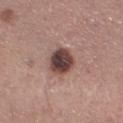biopsy status = catalogued during a skin exam; not biopsied | lesion diameter = about 3.5 mm | image-analysis metrics = border irregularity of about 1 on a 0–10 scale and peripheral color asymmetry of about 1.5; an automated nevus-likeness rating near 30 out of 100 and a detector confidence of about 100 out of 100 that the crop contains a lesion | site = the left lower leg | image = ~15 mm crop, total-body skin-cancer survey | tile lighting = white-light illumination | patient = female, aged around 30.